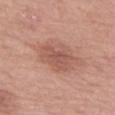This image is a 15 mm lesion crop taken from a total-body photograph. On the right forearm. The lesion-visualizer software estimated a mean CIELAB color near L≈55 a*≈23 b*≈27, a lesion–skin lightness drop of about 9, and a normalized lesion–skin contrast near 6. The software also gave a peripheral color-asymmetry measure near 1. The analysis additionally found a nevus-likeness score of about 30/100 and a lesion-detection confidence of about 100/100. Imaged with white-light lighting. A female patient aged 63 to 67. Measured at roughly 4.5 mm in maximum diameter.